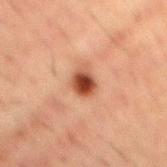Captured under cross-polarized illumination. Longest diameter approximately 3 mm. From the mid back. A male subject aged 48 to 52. Cropped from a total-body skin-imaging series; the visible field is about 15 mm.